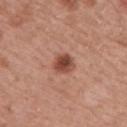On the upper back. Longest diameter approximately 2.5 mm. A 15 mm close-up tile from a total-body photography series done for melanoma screening. The subject is a male aged approximately 65. The tile uses white-light illumination.